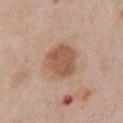<tbp_lesion>
  <biopsy_status>not biopsied; imaged during a skin examination</biopsy_status>
  <patient>
    <sex>male</sex>
    <age_approx>60</age_approx>
  </patient>
  <site>chest</site>
  <image>
    <source>total-body photography crop</source>
    <field_of_view_mm>15</field_of_view_mm>
  </image>
</tbp_lesion>